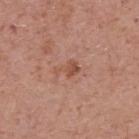notes — imaged on a skin check; not biopsied